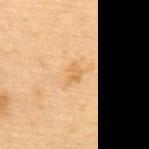A female patient approximately 65 years of age. The lesion-visualizer software estimated a footprint of about 3 mm² and an eccentricity of roughly 0.75. The analysis additionally found a lesion-to-skin contrast of about 5.5 (normalized; higher = more distinct). The analysis additionally found a border-irregularity index near 3.5/10, a within-lesion color-variation index near 2/10, and a peripheral color-asymmetry measure near 0.5. The tile uses cross-polarized illumination. Approximately 2.5 mm at its widest. Cropped from a whole-body photographic skin survey; the tile spans about 15 mm. Located on the upper back.